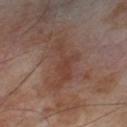On the leg. Approximately 7 mm at its widest. Cropped from a total-body skin-imaging series; the visible field is about 15 mm. A male subject, aged 68 to 72. Automated image analysis of the tile measured an eccentricity of roughly 0.9 and a shape-asymmetry score of about 0.6 (0 = symmetric). The software also gave an average lesion color of about L≈39 a*≈19 b*≈24 (CIELAB) and a normalized border contrast of about 6.5.